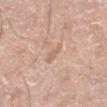{"biopsy_status": "not biopsied; imaged during a skin examination", "image": {"source": "total-body photography crop", "field_of_view_mm": 15}, "automated_metrics": {"shape_asymmetry": 0.35}, "patient": {"sex": "male", "age_approx": 60}, "site": "right lower leg"}